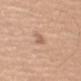Assessment: No biopsy was performed on this lesion — it was imaged during a full skin examination and was not determined to be concerning.